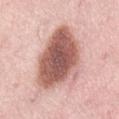Clinical impression: Recorded during total-body skin imaging; not selected for excision or biopsy. Context: An algorithmic analysis of the crop reported an outline eccentricity of about 0.75 (0 = round, 1 = elongated) and a symmetry-axis asymmetry near 0.25. The analysis additionally found a border-irregularity rating of about 2.5/10, a within-lesion color-variation index near 6.5/10, and radial color variation of about 2. Located on the lower back. About 8 mm across. The patient is a female roughly 50 years of age. This image is a 15 mm lesion crop taken from a total-body photograph. This is a white-light tile.